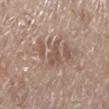Captured during whole-body skin photography for melanoma surveillance; the lesion was not biopsied.
A 15 mm close-up tile from a total-body photography series done for melanoma screening.
Measured at roughly 3 mm in maximum diameter.
This is a white-light tile.
The subject is a male in their 70s.
From the leg.